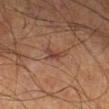  biopsy_status: not biopsied; imaged during a skin examination
  patient:
    sex: male
    age_approx: 65
  site: left lower leg
  lesion_size:
    long_diameter_mm_approx: 3.0
  image:
    source: total-body photography crop
    field_of_view_mm: 15
  lighting: cross-polarized